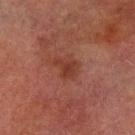No biopsy was performed on this lesion — it was imaged during a full skin examination and was not determined to be concerning.
Cropped from a whole-body photographic skin survey; the tile spans about 15 mm.
The lesion is on the left lower leg.
Captured under cross-polarized illumination.
The recorded lesion diameter is about 3 mm.
A male subject, aged 73–77.
An algorithmic analysis of the crop reported a lesion area of about 4.5 mm² and two-axis asymmetry of about 0.45. The software also gave an average lesion color of about L≈31 a*≈22 b*≈25 (CIELAB) and a normalized border contrast of about 6.5.